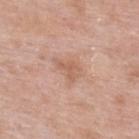Impression: Recorded during total-body skin imaging; not selected for excision or biopsy. Acquisition and patient details: The subject is a male aged 53 to 57. The recorded lesion diameter is about 3 mm. A region of skin cropped from a whole-body photographic capture, roughly 15 mm wide. This is a white-light tile. From the upper back.